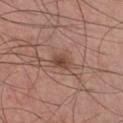<tbp_lesion>
  <biopsy_status>not biopsied; imaged during a skin examination</biopsy_status>
  <image>
    <source>total-body photography crop</source>
    <field_of_view_mm>15</field_of_view_mm>
  </image>
  <lesion_size>
    <long_diameter_mm_approx>2.5</long_diameter_mm_approx>
  </lesion_size>
  <lighting>white-light</lighting>
  <site>front of the torso</site>
  <patient>
    <sex>male</sex>
    <age_approx>30</age_approx>
  </patient>
</tbp_lesion>